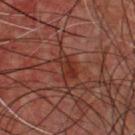<case>
  <biopsy_status>not biopsied; imaged during a skin examination</biopsy_status>
  <image>
    <source>total-body photography crop</source>
    <field_of_view_mm>15</field_of_view_mm>
  </image>
  <automated_metrics>
    <cielab_L>30</cielab_L>
    <cielab_a>25</cielab_a>
    <cielab_b>26</cielab_b>
    <vs_skin_darker_L>7.0</vs_skin_darker_L>
    <vs_skin_contrast_norm>7.0</vs_skin_contrast_norm>
  </automated_metrics>
  <site>chest</site>
  <patient>
    <sex>male</sex>
    <age_approx>45</age_approx>
  </patient>
  <lesion_size>
    <long_diameter_mm_approx>3.0</long_diameter_mm_approx>
  </lesion_size>
  <lighting>cross-polarized</lighting>
</case>